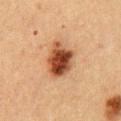workup = catalogued during a skin exam; not biopsied
lesion size = about 5 mm
image = ~15 mm tile from a whole-body skin photo
patient = male, aged 48–52
image-analysis metrics = a lesion color around L≈40 a*≈21 b*≈30 in CIELAB; a lesion-detection confidence of about 100/100
anatomic site = the chest